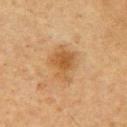biopsy_status: not biopsied; imaged during a skin examination
image:
  source: total-body photography crop
  field_of_view_mm: 15
site: right upper arm
lighting: cross-polarized
automated_metrics:
  area_mm2_approx: 11.0
  eccentricity: 0.65
  shape_asymmetry: 0.3
  peripheral_color_asymmetry: 1.0
  nevus_likeness_0_100: 65
  lesion_detection_confidence_0_100: 100
patient:
  sex: male
  age_approx: 65